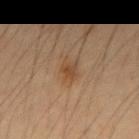Impression:
Part of a total-body skin-imaging series; this lesion was reviewed on a skin check and was not flagged for biopsy.
Acquisition and patient details:
On the left forearm. Longest diameter approximately 3 mm. A region of skin cropped from a whole-body photographic capture, roughly 15 mm wide. A male patient aged 33 to 37. This is a cross-polarized tile.